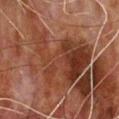Findings:
- follow-up: total-body-photography surveillance lesion; no biopsy
- image source: ~15 mm crop, total-body skin-cancer survey
- image-analysis metrics: an eccentricity of roughly 0.6 and a symmetry-axis asymmetry near 0.4; a mean CIELAB color near L≈30 a*≈20 b*≈25, a lesion–skin lightness drop of about 7, and a normalized border contrast of about 7.5
- location: the chest
- subject: male, aged around 65